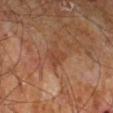{"biopsy_status": "not biopsied; imaged during a skin examination", "patient": {"sex": "male", "age_approx": 60}, "lesion_size": {"long_diameter_mm_approx": 2.5}, "site": "right leg", "lighting": "cross-polarized", "image": {"source": "total-body photography crop", "field_of_view_mm": 15}}